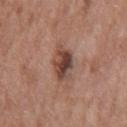<record>
<site>mid back</site>
<lesion_size>
  <long_diameter_mm_approx>4.0</long_diameter_mm_approx>
</lesion_size>
<image>
  <source>total-body photography crop</source>
  <field_of_view_mm>15</field_of_view_mm>
</image>
<lighting>white-light</lighting>
<patient>
  <sex>male</sex>
  <age_approx>50</age_approx>
</patient>
</record>